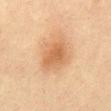<case>
  <biopsy_status>not biopsied; imaged during a skin examination</biopsy_status>
  <patient>
    <sex>female</sex>
    <age_approx>55</age_approx>
  </patient>
  <site>front of the torso</site>
  <image>
    <source>total-body photography crop</source>
    <field_of_view_mm>15</field_of_view_mm>
  </image>
  <automated_metrics>
    <area_mm2_approx>9.0</area_mm2_approx>
    <eccentricity>0.75</eccentricity>
    <shape_asymmetry>0.2</shape_asymmetry>
    <cielab_L>54</cielab_L>
    <cielab_a>20</cielab_a>
    <cielab_b>35</cielab_b>
    <vs_skin_darker_L>8.0</vs_skin_darker_L>
    <vs_skin_contrast_norm>6.5</vs_skin_contrast_norm>
    <border_irregularity_0_10>2.0</border_irregularity_0_10>
    <color_variation_0_10>2.5</color_variation_0_10>
    <nevus_likeness_0_100>45</nevus_likeness_0_100>
    <lesion_detection_confidence_0_100>100</lesion_detection_confidence_0_100>
  </automated_metrics>
</case>